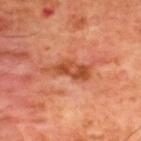Part of a total-body skin-imaging series; this lesion was reviewed on a skin check and was not flagged for biopsy.
The patient is a male roughly 60 years of age.
A lesion tile, about 15 mm wide, cut from a 3D total-body photograph.
On the upper back.